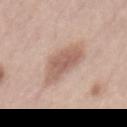workup: total-body-photography surveillance lesion; no biopsy.